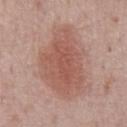Notes:
– biopsy status: total-body-photography surveillance lesion; no biopsy
– automated lesion analysis: an average lesion color of about L≈54 a*≈23 b*≈26 (CIELAB), about 9 CIELAB-L* units darker than the surrounding skin, and a lesion-to-skin contrast of about 6.5 (normalized; higher = more distinct); a nevus-likeness score of about 90/100 and a detector confidence of about 100 out of 100 that the crop contains a lesion
– location: the front of the torso
– acquisition: total-body-photography crop, ~15 mm field of view
– subject: male, aged approximately 75
– size: about 7.5 mm
– lighting: white-light illumination